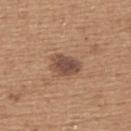The lesion's longest dimension is about 3.5 mm.
Cropped from a whole-body photographic skin survey; the tile spans about 15 mm.
Automated image analysis of the tile measured a lesion color around L≈48 a*≈19 b*≈27 in CIELAB and a lesion–skin lightness drop of about 12. It also reported a lesion-detection confidence of about 100/100.
From the upper back.
Captured under white-light illumination.
A male patient, about 65 years old.